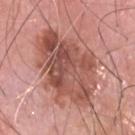No biopsy was performed on this lesion — it was imaged during a full skin examination and was not determined to be concerning. An algorithmic analysis of the crop reported an average lesion color of about L≈52 a*≈25 b*≈27 (CIELAB), about 13 CIELAB-L* units darker than the surrounding skin, and a normalized border contrast of about 8.5. And it measured a border-irregularity index near 4.5/10, internal color variation of about 8 on a 0–10 scale, and radial color variation of about 3. The analysis additionally found a classifier nevus-likeness of about 10/100 and a detector confidence of about 100 out of 100 that the crop contains a lesion. The lesion is located on the head or neck. A 15 mm crop from a total-body photograph taken for skin-cancer surveillance. The subject is a male aged approximately 80. The recorded lesion diameter is about 9.5 mm. Imaged with white-light lighting.